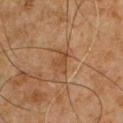Q: What is the lesion's diameter?
A: about 3 mm
Q: Lesion location?
A: the chest
Q: What is the imaging modality?
A: ~15 mm crop, total-body skin-cancer survey
Q: How was the tile lit?
A: cross-polarized illumination
Q: Automated lesion metrics?
A: a lesion area of about 4.5 mm², an eccentricity of roughly 0.8, and two-axis asymmetry of about 0.4
Q: What are the patient's age and sex?
A: male, approximately 60 years of age